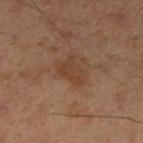No biopsy was performed on this lesion — it was imaged during a full skin examination and was not determined to be concerning. About 3.5 mm across. A 15 mm close-up tile from a total-body photography series done for melanoma screening. A male patient, aged approximately 50. The lesion is on the right lower leg.